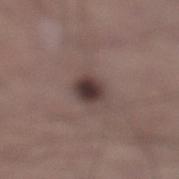From the left lower leg.
A lesion tile, about 15 mm wide, cut from a 3D total-body photograph.
The patient is a male in their mid-30s.
The total-body-photography lesion software estimated an area of roughly 5.5 mm², an eccentricity of roughly 0.5, and a symmetry-axis asymmetry near 0.15. And it measured a within-lesion color-variation index near 4.5/10 and a peripheral color-asymmetry measure near 1.
The recorded lesion diameter is about 3 mm.
This is a white-light tile.